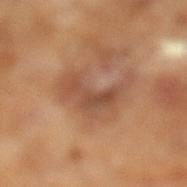The lesion was photographed on a routine skin check and not biopsied; there is no pathology result. Longest diameter approximately 5.5 mm. A male subject, aged around 65. The lesion-visualizer software estimated an automated nevus-likeness rating near 0 out of 100 and a lesion-detection confidence of about 100/100. Captured under cross-polarized illumination. Cropped from a whole-body photographic skin survey; the tile spans about 15 mm.